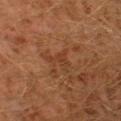A close-up tile cropped from a whole-body skin photograph, about 15 mm across. The tile uses cross-polarized illumination. Approximately 3 mm at its widest. A male subject aged approximately 30. Located on the left thigh.